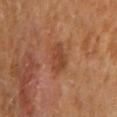A region of skin cropped from a whole-body photographic capture, roughly 15 mm wide. From the mid back. A male subject aged 63 to 67.